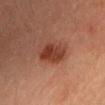{"biopsy_status": "not biopsied; imaged during a skin examination", "image": {"source": "total-body photography crop", "field_of_view_mm": 15}, "lighting": "cross-polarized", "automated_metrics": {"area_mm2_approx": 8.5, "eccentricity": 0.6, "shape_asymmetry": 0.2, "nevus_likeness_0_100": 100, "lesion_detection_confidence_0_100": 100}, "patient": {"sex": "female", "age_approx": 35}, "site": "head or neck"}